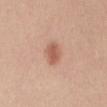This lesion was catalogued during total-body skin photography and was not selected for biopsy. A 15 mm close-up extracted from a 3D total-body photography capture. The recorded lesion diameter is about 3 mm. From the mid back. Imaged with white-light lighting. A female subject, in their mid- to late 40s.